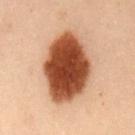Assessment:
Part of a total-body skin-imaging series; this lesion was reviewed on a skin check and was not flagged for biopsy.
Clinical summary:
This image is a 15 mm lesion crop taken from a total-body photograph. The lesion-visualizer software estimated an outline eccentricity of about 0.75 (0 = round, 1 = elongated) and a symmetry-axis asymmetry near 0.1. It also reported a border-irregularity index near 1.5/10. The lesion is on the mid back. Imaged with cross-polarized lighting. Measured at roughly 8 mm in maximum diameter. A male patient aged approximately 55.